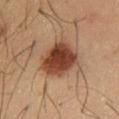This lesion was catalogued during total-body skin photography and was not selected for biopsy.
The patient is a male aged around 40.
On the chest.
This image is a 15 mm lesion crop taken from a total-body photograph.
The tile uses cross-polarized illumination.